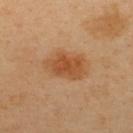biopsy status: catalogued during a skin exam; not biopsied | patient: male, aged approximately 40 | tile lighting: cross-polarized illumination | image: total-body-photography crop, ~15 mm field of view | lesion diameter: about 5 mm | automated metrics: an average lesion color of about L≈51 a*≈23 b*≈39 (CIELAB), roughly 10 lightness units darker than nearby skin, and a lesion-to-skin contrast of about 8 (normalized; higher = more distinct); internal color variation of about 3 on a 0–10 scale and radial color variation of about 1; a nevus-likeness score of about 100/100 | anatomic site: the back.